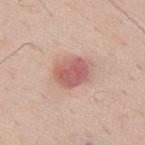No biopsy was performed on this lesion — it was imaged during a full skin examination and was not determined to be concerning. A 15 mm close-up extracted from a 3D total-body photography capture. Located on the upper back. An algorithmic analysis of the crop reported a lesion area of about 10 mm² and a shape eccentricity near 0.65. And it measured a lesion color around L≈59 a*≈24 b*≈25 in CIELAB and a normalized border contrast of about 8. And it measured border irregularity of about 1.5 on a 0–10 scale, internal color variation of about 4 on a 0–10 scale, and peripheral color asymmetry of about 1.5. A male subject, in their mid- to late 50s. The lesion's longest dimension is about 4 mm.